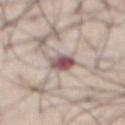Imaged during a routine full-body skin examination; the lesion was not biopsied and no histopathology is available.
The recorded lesion diameter is about 3 mm.
Imaged with white-light lighting.
Automated image analysis of the tile measured a border-irregularity index near 2/10 and internal color variation of about 7.5 on a 0–10 scale. It also reported a classifier nevus-likeness of about 20/100 and a lesion-detection confidence of about 100/100.
From the abdomen.
A roughly 15 mm field-of-view crop from a total-body skin photograph.
A male patient aged 68 to 72.